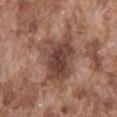Findings:
– follow-up — imaged on a skin check; not biopsied
– patient — male, aged around 75
– acquisition — 15 mm crop, total-body photography
– site — the abdomen
– lesion diameter — ≈6.5 mm